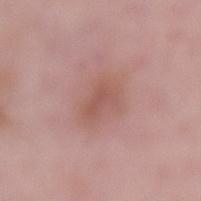| key | value |
|---|---|
| image source | ~15 mm crop, total-body skin-cancer survey |
| diameter | about 3 mm |
| lighting | white-light |
| site | the lower back |
| patient | male, aged 53 to 57 |